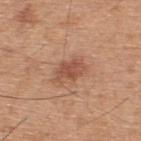| field | value |
|---|---|
| follow-up | catalogued during a skin exam; not biopsied |
| image-analysis metrics | an eccentricity of roughly 0.8; a detector confidence of about 100 out of 100 that the crop contains a lesion |
| lesion diameter | about 4 mm |
| image | ~15 mm tile from a whole-body skin photo |
| patient | male, aged approximately 45 |
| anatomic site | the upper back |
| lighting | white-light illumination |